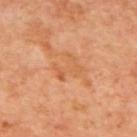biopsy status: catalogued during a skin exam; not biopsied | illumination: cross-polarized | diameter: ~3 mm (longest diameter) | body site: the back | image: 15 mm crop, total-body photography | patient: roughly 65 years of age.